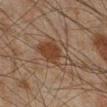Captured during whole-body skin photography for melanoma surveillance; the lesion was not biopsied.
Imaged with cross-polarized lighting.
A male subject approximately 45 years of age.
Measured at roughly 5.5 mm in maximum diameter.
The lesion is located on the right lower leg.
A close-up tile cropped from a whole-body skin photograph, about 15 mm across.